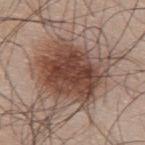Impression: Captured during whole-body skin photography for melanoma surveillance; the lesion was not biopsied. Acquisition and patient details: From the upper back. This image is a 15 mm lesion crop taken from a total-body photograph. Approximately 8 mm at its widest. The subject is a male in their mid- to late 40s. This is a white-light tile.